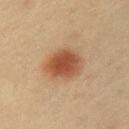Notes:
• follow-up · total-body-photography surveillance lesion; no biopsy